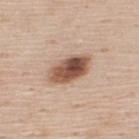Captured during whole-body skin photography for melanoma surveillance; the lesion was not biopsied. The lesion is on the back. A 15 mm close-up tile from a total-body photography series done for melanoma screening. The recorded lesion diameter is about 5 mm. Captured under white-light illumination. The patient is a male approximately 45 years of age. Automated image analysis of the tile measured an area of roughly 12 mm². It also reported border irregularity of about 1.5 on a 0–10 scale and a color-variation rating of about 8.5/10. The analysis additionally found a nevus-likeness score of about 95/100.